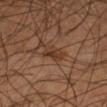Notes:
– location · the right lower leg
– image · ~15 mm tile from a whole-body skin photo
– subject · male, approximately 55 years of age
– tile lighting · cross-polarized illumination
– lesion size · about 3 mm
– image-analysis metrics · an outline eccentricity of about 0.75 (0 = round, 1 = elongated) and a shape-asymmetry score of about 0.25 (0 = symmetric)